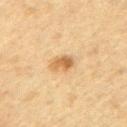Assessment:
The lesion was tiled from a total-body skin photograph and was not biopsied.
Image and clinical context:
The lesion's longest dimension is about 2.5 mm. The tile uses cross-polarized illumination. Cropped from a whole-body photographic skin survey; the tile spans about 15 mm. A male subject, aged around 60. The total-body-photography lesion software estimated a lesion area of about 4.5 mm², a shape eccentricity near 0.8, and a shape-asymmetry score of about 0.3 (0 = symmetric). The software also gave a mean CIELAB color near L≈56 a*≈18 b*≈38 and a lesion-to-skin contrast of about 8 (normalized; higher = more distinct). And it measured a border-irregularity index near 3/10, a within-lesion color-variation index near 3.5/10, and radial color variation of about 1.